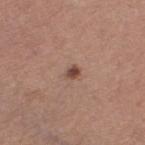Q: Was this lesion biopsied?
A: total-body-photography surveillance lesion; no biopsy
Q: What is the lesion's diameter?
A: ~1.5 mm (longest diameter)
Q: What are the patient's age and sex?
A: female, approximately 60 years of age
Q: What is the imaging modality?
A: 15 mm crop, total-body photography
Q: Where on the body is the lesion?
A: the left thigh